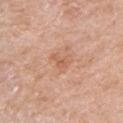Assessment: Imaged during a routine full-body skin examination; the lesion was not biopsied and no histopathology is available. Clinical summary: The tile uses white-light illumination. The lesion is located on the right upper arm. A close-up tile cropped from a whole-body skin photograph, about 15 mm across. About 2.5 mm across. The patient is a female in their mid- to late 50s.